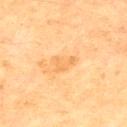notes: no biopsy performed (imaged during a skin exam); body site: the chest; patient: male, aged approximately 65; image source: 15 mm crop, total-body photography.